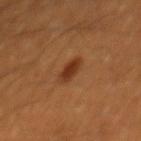Q: Was a biopsy performed?
A: imaged on a skin check; not biopsied
Q: What is the anatomic site?
A: the mid back
Q: What are the patient's age and sex?
A: male, roughly 60 years of age
Q: How large is the lesion?
A: about 3 mm
Q: Illumination type?
A: cross-polarized illumination
Q: What kind of image is this?
A: total-body-photography crop, ~15 mm field of view
Q: What did automated image analysis measure?
A: about 10 CIELAB-L* units darker than the surrounding skin and a normalized lesion–skin contrast near 9; border irregularity of about 2.5 on a 0–10 scale, a color-variation rating of about 1.5/10, and a peripheral color-asymmetry measure near 0.5; a classifier nevus-likeness of about 100/100 and a lesion-detection confidence of about 100/100